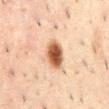Q: Was a biopsy performed?
A: imaged on a skin check; not biopsied
Q: Who is the patient?
A: male, in their 50s
Q: What lighting was used for the tile?
A: cross-polarized illumination
Q: What did automated image analysis measure?
A: about 16 CIELAB-L* units darker than the surrounding skin and a normalized lesion–skin contrast near 12; a detector confidence of about 100 out of 100 that the crop contains a lesion
Q: How large is the lesion?
A: ≈3.5 mm
Q: Lesion location?
A: the abdomen
Q: What is the imaging modality?
A: ~15 mm crop, total-body skin-cancer survey